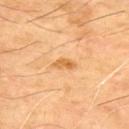Captured during whole-body skin photography for melanoma surveillance; the lesion was not biopsied. The subject is a male in their mid-60s. A region of skin cropped from a whole-body photographic capture, roughly 15 mm wide. The lesion-visualizer software estimated an average lesion color of about L≈63 a*≈23 b*≈46 (CIELAB) and a lesion-to-skin contrast of about 7 (normalized; higher = more distinct). On the upper back.